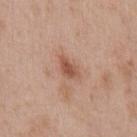Imaged during a routine full-body skin examination; the lesion was not biopsied and no histopathology is available. Located on the chest. A male patient aged 63–67. About 3 mm across. The tile uses white-light illumination. A 15 mm crop from a total-body photograph taken for skin-cancer surveillance.